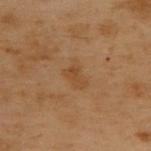No biopsy was performed on this lesion — it was imaged during a full skin examination and was not determined to be concerning.
A 15 mm crop from a total-body photograph taken for skin-cancer surveillance.
A male patient, approximately 55 years of age.
This is a cross-polarized tile.
Located on the back.